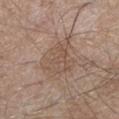Assessment: No biopsy was performed on this lesion — it was imaged during a full skin examination and was not determined to be concerning. Acquisition and patient details: About 5 mm across. Cropped from a total-body skin-imaging series; the visible field is about 15 mm. A male patient aged 58 to 62. Automated image analysis of the tile measured a border-irregularity rating of about 3/10, a color-variation rating of about 3/10, and peripheral color asymmetry of about 1. The analysis additionally found an automated nevus-likeness rating near 0 out of 100 and a lesion-detection confidence of about 100/100. The lesion is located on the left lower leg.